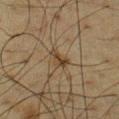| field | value |
|---|---|
| notes | total-body-photography surveillance lesion; no biopsy |
| image source | total-body-photography crop, ~15 mm field of view |
| tile lighting | cross-polarized illumination |
| body site | the chest |
| patient | male, about 60 years old |
| size | ≈2.5 mm |